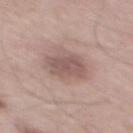Q: How was this image acquired?
A: ~15 mm tile from a whole-body skin photo
Q: Who is the patient?
A: male, aged 53 to 57
Q: Lesion location?
A: the mid back
Q: What did automated image analysis measure?
A: an area of roughly 10 mm², an outline eccentricity of about 0.55 (0 = round, 1 = elongated), and a symmetry-axis asymmetry near 0.15; a mean CIELAB color near L≈54 a*≈18 b*≈21, about 9 CIELAB-L* units darker than the surrounding skin, and a normalized lesion–skin contrast near 6.5; lesion-presence confidence of about 100/100
Q: What is the lesion's diameter?
A: ~3.5 mm (longest diameter)
Q: What lighting was used for the tile?
A: white-light illumination